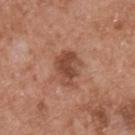The subject is a male in their mid- to late 50s.
The tile uses white-light illumination.
The lesion-visualizer software estimated a lesion area of about 9 mm², an outline eccentricity of about 0.65 (0 = round, 1 = elongated), and two-axis asymmetry of about 0.3. The analysis additionally found an average lesion color of about L≈47 a*≈24 b*≈30 (CIELAB), about 11 CIELAB-L* units darker than the surrounding skin, and a lesion-to-skin contrast of about 8 (normalized; higher = more distinct). It also reported internal color variation of about 4 on a 0–10 scale and radial color variation of about 1.5.
On the upper back.
About 4 mm across.
Cropped from a total-body skin-imaging series; the visible field is about 15 mm.